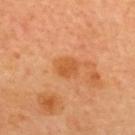<tbp_lesion>
<biopsy_status>not biopsied; imaged during a skin examination</biopsy_status>
<lesion_size>
  <long_diameter_mm_approx>2.5</long_diameter_mm_approx>
</lesion_size>
<automated_metrics>
  <border_irregularity_0_10>1.5</border_irregularity_0_10>
  <color_variation_0_10>1.5</color_variation_0_10>
  <peripheral_color_asymmetry>0.5</peripheral_color_asymmetry>
</automated_metrics>
<patient>
  <sex>male</sex>
  <age_approx>65</age_approx>
</patient>
<image>
  <source>total-body photography crop</source>
  <field_of_view_mm>15</field_of_view_mm>
</image>
<site>upper back</site>
</tbp_lesion>